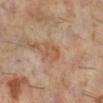Clinical impression:
No biopsy was performed on this lesion — it was imaged during a full skin examination and was not determined to be concerning.
Clinical summary:
The patient is a female roughly 60 years of age. Located on the right lower leg. About 3 mm across. The tile uses cross-polarized illumination. This image is a 15 mm lesion crop taken from a total-body photograph.